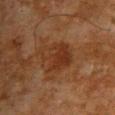Imaged during a routine full-body skin examination; the lesion was not biopsied and no histopathology is available. A male patient, aged 63 to 67. Automated tile analysis of the lesion measured a lesion color around L≈26 a*≈19 b*≈26 in CIELAB. The analysis additionally found a border-irregularity rating of about 3.5/10, a color-variation rating of about 4.5/10, and radial color variation of about 1.5. The analysis additionally found lesion-presence confidence of about 100/100. Located on the chest. Cropped from a total-body skin-imaging series; the visible field is about 15 mm. The recorded lesion diameter is about 4.5 mm.